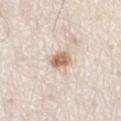The lesion is located on the front of the torso. The tile uses white-light illumination. A region of skin cropped from a whole-body photographic capture, roughly 15 mm wide. Automated image analysis of the tile measured roughly 14 lightness units darker than nearby skin and a normalized border contrast of about 9. The analysis additionally found a border-irregularity rating of about 1.5/10, a color-variation rating of about 4.5/10, and a peripheral color-asymmetry measure near 1.5. The software also gave a classifier nevus-likeness of about 100/100 and a detector confidence of about 100 out of 100 that the crop contains a lesion. A male subject aged approximately 80. About 3 mm across.